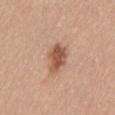Findings:
* notes: total-body-photography surveillance lesion; no biopsy
* body site: the back
* imaging modality: ~15 mm tile from a whole-body skin photo
* lighting: white-light
* patient: female, about 40 years old